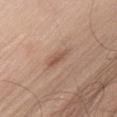Imaged during a routine full-body skin examination; the lesion was not biopsied and no histopathology is available. This image is a 15 mm lesion crop taken from a total-body photograph. From the left upper arm. A male subject roughly 55 years of age.